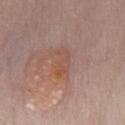Assessment: Recorded during total-body skin imaging; not selected for excision or biopsy. Acquisition and patient details: A close-up tile cropped from a whole-body skin photograph, about 15 mm across. Imaged with white-light lighting. An algorithmic analysis of the crop reported a mean CIELAB color near L≈52 a*≈21 b*≈28, roughly 5 lightness units darker than nearby skin, and a normalized border contrast of about 5.5. The analysis additionally found a lesion-detection confidence of about 100/100. A male subject aged 83 or older. Located on the chest. About 4 mm across.